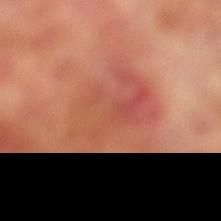The lesion was tiled from a total-body skin photograph and was not biopsied. The lesion is on the left lower leg. A male patient, aged around 70. Measured at roughly 7 mm in maximum diameter. A region of skin cropped from a whole-body photographic capture, roughly 15 mm wide.